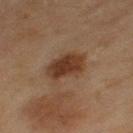notes: catalogued during a skin exam; not biopsied | patient: female, aged approximately 60 | body site: the left thigh | image: total-body-photography crop, ~15 mm field of view | size: ≈4.5 mm | TBP lesion metrics: a nevus-likeness score of about 90/100 and lesion-presence confidence of about 100/100 | tile lighting: cross-polarized illumination.